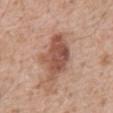| feature | finding |
|---|---|
| follow-up | no biopsy performed (imaged during a skin exam) |